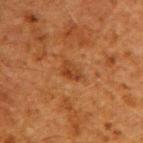Recorded during total-body skin imaging; not selected for excision or biopsy. Measured at roughly 2.5 mm in maximum diameter. From the right upper arm. A male subject, about 60 years old. The tile uses cross-polarized illumination. Cropped from a total-body skin-imaging series; the visible field is about 15 mm. The lesion-visualizer software estimated a footprint of about 3.5 mm², an outline eccentricity of about 0.8 (0 = round, 1 = elongated), and a shape-asymmetry score of about 0.25 (0 = symmetric). It also reported an average lesion color of about L≈32 a*≈22 b*≈32 (CIELAB), roughly 7 lightness units darker than nearby skin, and a normalized border contrast of about 6.5.